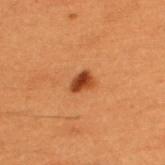The lesion was tiled from a total-body skin photograph and was not biopsied. The subject is a male roughly 50 years of age. A lesion tile, about 15 mm wide, cut from a 3D total-body photograph. The lesion is on the upper back. Approximately 2.5 mm at its widest. The tile uses cross-polarized illumination.